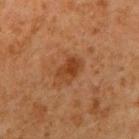Captured during whole-body skin photography for melanoma surveillance; the lesion was not biopsied. Cropped from a whole-body photographic skin survey; the tile spans about 15 mm. The recorded lesion diameter is about 3 mm. The total-body-photography lesion software estimated a mean CIELAB color near L≈34 a*≈22 b*≈32 and roughly 8 lightness units darker than nearby skin. The patient is a male approximately 60 years of age. On the right upper arm. Captured under cross-polarized illumination.